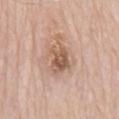Clinical impression:
Part of a total-body skin-imaging series; this lesion was reviewed on a skin check and was not flagged for biopsy.
Clinical summary:
Cropped from a whole-body photographic skin survey; the tile spans about 15 mm. A male subject, in their mid-80s or older. About 3.5 mm across. The lesion-visualizer software estimated a lesion area of about 7.5 mm², an outline eccentricity of about 0.65 (0 = round, 1 = elongated), and a shape-asymmetry score of about 0.2 (0 = symmetric). The software also gave border irregularity of about 2.5 on a 0–10 scale, internal color variation of about 4.5 on a 0–10 scale, and radial color variation of about 1.5. The analysis additionally found a classifier nevus-likeness of about 0/100 and lesion-presence confidence of about 100/100. On the mid back. Imaged with white-light lighting.